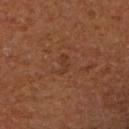Background: A female subject. The lesion-visualizer software estimated a footprint of about 2.5 mm², an outline eccentricity of about 0.9 (0 = round, 1 = elongated), and two-axis asymmetry of about 0.45. And it measured an average lesion color of about L≈37 a*≈23 b*≈31 (CIELAB), roughly 5 lightness units darker than nearby skin, and a normalized border contrast of about 4.5. The analysis additionally found a border-irregularity rating of about 4.5/10 and internal color variation of about 0 on a 0–10 scale. A region of skin cropped from a whole-body photographic capture, roughly 15 mm wide. The tile uses cross-polarized illumination. On the left upper arm.